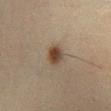Q: Was this lesion biopsied?
A: no biopsy performed (imaged during a skin exam)
Q: Lesion location?
A: the right lower leg
Q: What are the patient's age and sex?
A: male, aged approximately 30
Q: What is the imaging modality?
A: 15 mm crop, total-body photography